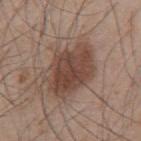Notes:
- biopsy status: no biopsy performed (imaged during a skin exam)
- anatomic site: the mid back
- illumination: white-light
- lesion diameter: ≈6.5 mm
- patient: male, aged approximately 55
- imaging modality: ~15 mm tile from a whole-body skin photo